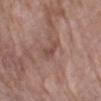Clinical impression:
No biopsy was performed on this lesion — it was imaged during a full skin examination and was not determined to be concerning.
Background:
The subject is a female aged 68 to 72. A 15 mm crop from a total-body photograph taken for skin-cancer surveillance. From the left forearm.